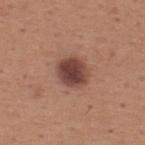The lesion was photographed on a routine skin check and not biopsied; there is no pathology result.
From the back.
A roughly 15 mm field-of-view crop from a total-body skin photograph.
The total-body-photography lesion software estimated an area of roughly 9 mm², an outline eccentricity of about 0.55 (0 = round, 1 = elongated), and a shape-asymmetry score of about 0.1 (0 = symmetric).
The subject is a male approximately 65 years of age.
The recorded lesion diameter is about 4 mm.